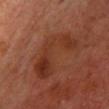– notes — no biopsy performed (imaged during a skin exam)
– subject — male, about 60 years old
– imaging modality — total-body-photography crop, ~15 mm field of view
– site — the upper back